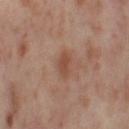Findings:
- biopsy status · catalogued during a skin exam; not biopsied
- site · the right thigh
- image source · ~15 mm tile from a whole-body skin photo
- subject · female, in their mid- to late 50s
- lesion diameter · ~2.5 mm (longest diameter)
- tile lighting · cross-polarized